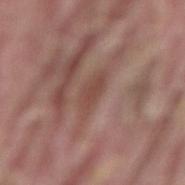{"biopsy_status": "not biopsied; imaged during a skin examination", "image": {"source": "total-body photography crop", "field_of_view_mm": 15}, "automated_metrics": {"peripheral_color_asymmetry": 0.5, "nevus_likeness_0_100": 0, "lesion_detection_confidence_0_100": 80}, "site": "back", "lighting": "white-light", "patient": {"sex": "male", "age_approx": 40}, "lesion_size": {"long_diameter_mm_approx": 4.0}}